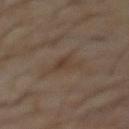No biopsy was performed on this lesion — it was imaged during a full skin examination and was not determined to be concerning.
A male subject aged around 60.
The lesion's longest dimension is about 3.5 mm.
Automated image analysis of the tile measured two-axis asymmetry of about 0.55. The software also gave a classifier nevus-likeness of about 0/100 and a detector confidence of about 100 out of 100 that the crop contains a lesion.
From the front of the torso.
Cropped from a total-body skin-imaging series; the visible field is about 15 mm.